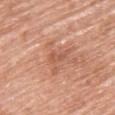Captured during whole-body skin photography for melanoma surveillance; the lesion was not biopsied. Captured under white-light illumination. On the upper back. The lesion's longest dimension is about 2.5 mm. A female patient, aged around 60. A lesion tile, about 15 mm wide, cut from a 3D total-body photograph.